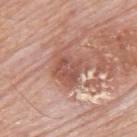| feature | finding |
|---|---|
| biopsy status | no biopsy performed (imaged during a skin exam) |
| anatomic site | the back |
| image source | ~15 mm crop, total-body skin-cancer survey |
| subject | male, approximately 80 years of age |